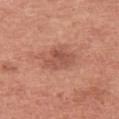The lesion was tiled from a total-body skin photograph and was not biopsied.
The lesion is on the left upper arm.
Approximately 3.5 mm at its widest.
A lesion tile, about 15 mm wide, cut from a 3D total-body photograph.
A female patient, in their 50s.
Captured under white-light illumination.